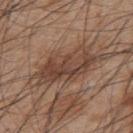biopsy status: no biopsy performed (imaged during a skin exam)
patient: male, aged approximately 45
image source: 15 mm crop, total-body photography
anatomic site: the upper back
TBP lesion metrics: an area of roughly 18 mm², an eccentricity of roughly 0.9, and two-axis asymmetry of about 0.3